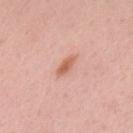follow-up — total-body-photography surveillance lesion; no biopsy
lighting — white-light illumination
lesion size — about 3 mm
patient — male, in their 50s
automated metrics — a mean CIELAB color near L≈63 a*≈25 b*≈31, a lesion–skin lightness drop of about 10, and a normalized lesion–skin contrast near 7.5; a border-irregularity rating of about 2.5/10 and a within-lesion color-variation index near 2.5/10; a nevus-likeness score of about 80/100 and lesion-presence confidence of about 100/100
image source — total-body-photography crop, ~15 mm field of view
location — the upper back